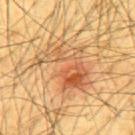biopsy status: no biopsy performed (imaged during a skin exam) | automated metrics: a mean CIELAB color near L≈63 a*≈23 b*≈42, a lesion–skin lightness drop of about 10, and a lesion-to-skin contrast of about 6.5 (normalized; higher = more distinct) | illumination: cross-polarized | image source: ~15 mm tile from a whole-body skin photo | subject: male, in their mid- to late 60s | body site: the back | diameter: about 7 mm.